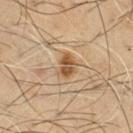Assessment: The lesion was tiled from a total-body skin photograph and was not biopsied. Clinical summary: The subject is a male in their mid-50s. Cropped from a total-body skin-imaging series; the visible field is about 15 mm. From the chest. Approximately 3 mm at its widest. The tile uses cross-polarized illumination.